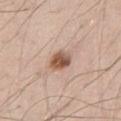Recorded during total-body skin imaging; not selected for excision or biopsy.
On the chest.
This is a white-light tile.
This image is a 15 mm lesion crop taken from a total-body photograph.
The patient is a male in their mid-40s.
The lesion-visualizer software estimated an eccentricity of roughly 0.65 and a symmetry-axis asymmetry near 0.2. And it measured a lesion color around L≈55 a*≈20 b*≈30 in CIELAB, a lesion–skin lightness drop of about 15, and a normalized lesion–skin contrast near 10. The analysis additionally found a detector confidence of about 100 out of 100 that the crop contains a lesion.
Measured at roughly 3 mm in maximum diameter.